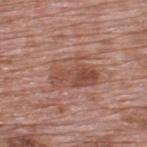Located on the upper back. A male patient aged 68 to 72. Cropped from a total-body skin-imaging series; the visible field is about 15 mm. The lesion-visualizer software estimated a lesion color around L≈50 a*≈22 b*≈27 in CIELAB and a normalized border contrast of about 6.5. The analysis additionally found a lesion-detection confidence of about 100/100. The lesion's longest dimension is about 5 mm. Captured under white-light illumination.